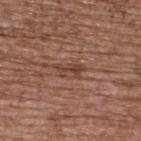The total-body-photography lesion software estimated border irregularity of about 6 on a 0–10 scale, internal color variation of about 0 on a 0–10 scale, and a peripheral color-asymmetry measure near 0. The lesion is on the upper back. Captured under white-light illumination. A female subject aged 63–67. Cropped from a whole-body photographic skin survey; the tile spans about 15 mm.